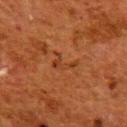Clinical impression:
The lesion was photographed on a routine skin check and not biopsied; there is no pathology result.
Image and clinical context:
Cropped from a whole-body photographic skin survey; the tile spans about 15 mm. A female patient, roughly 50 years of age. This is a cross-polarized tile. About 3 mm across. The total-body-photography lesion software estimated a lesion area of about 3.5 mm² and a shape-asymmetry score of about 0.7 (0 = symmetric). The software also gave a mean CIELAB color near L≈32 a*≈24 b*≈33 and about 6 CIELAB-L* units darker than the surrounding skin. Located on the upper back.